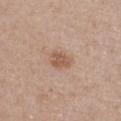biopsy status = total-body-photography surveillance lesion; no biopsy | subject = male, aged approximately 55 | site = the chest | image = 15 mm crop, total-body photography | size = about 2.5 mm.